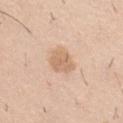workup: no biopsy performed (imaged during a skin exam) | image source: ~15 mm crop, total-body skin-cancer survey | lighting: white-light illumination | patient: male, aged 38–42 | anatomic site: the right thigh.